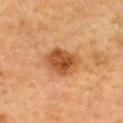Assessment: Imaged during a routine full-body skin examination; the lesion was not biopsied and no histopathology is available. Image and clinical context: A female patient aged approximately 60. An algorithmic analysis of the crop reported an outline eccentricity of about 0.65 (0 = round, 1 = elongated). It also reported an average lesion color of about L≈46 a*≈24 b*≈37 (CIELAB), about 12 CIELAB-L* units darker than the surrounding skin, and a normalized border contrast of about 9.5. The software also gave a nevus-likeness score of about 95/100 and lesion-presence confidence of about 100/100. On the upper back. Captured under cross-polarized illumination. A 15 mm close-up tile from a total-body photography series done for melanoma screening. Measured at roughly 4.5 mm in maximum diameter.